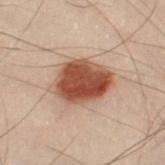biopsy status: total-body-photography surveillance lesion; no biopsy